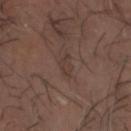– workup · total-body-photography surveillance lesion; no biopsy
– lighting · cross-polarized
– subject · male, approximately 65 years of age
– image · ~15 mm crop, total-body skin-cancer survey
– automated lesion analysis · a lesion area of about 3 mm² and an eccentricity of roughly 0.8; roughly 5 lightness units darker than nearby skin
– location · the head or neck